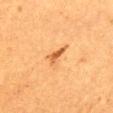Imaged during a routine full-body skin examination; the lesion was not biopsied and no histopathology is available. Imaged with cross-polarized lighting. A region of skin cropped from a whole-body photographic capture, roughly 15 mm wide. A female subject, in their mid-50s. The recorded lesion diameter is about 3 mm. On the back.